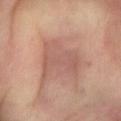lighting: cross-polarized
site: right forearm
patient:
  sex: male
  age_approx: 65
lesion_size:
  long_diameter_mm_approx: 6.5
image:
  source: total-body photography crop
  field_of_view_mm: 15